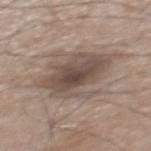| field | value |
|---|---|
| biopsy status | catalogued during a skin exam; not biopsied |
| imaging modality | total-body-photography crop, ~15 mm field of view |
| subject | male, roughly 75 years of age |
| site | the mid back |
| illumination | white-light |
| diameter | about 7 mm |